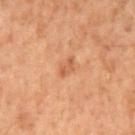Clinical impression: Part of a total-body skin-imaging series; this lesion was reviewed on a skin check and was not flagged for biopsy. Acquisition and patient details: Located on the mid back. A male subject, in their 70s. About 2.5 mm across. A 15 mm close-up extracted from a 3D total-body photography capture.